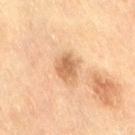Clinical impression: The lesion was photographed on a routine skin check and not biopsied; there is no pathology result. Acquisition and patient details: A region of skin cropped from a whole-body photographic capture, roughly 15 mm wide. An algorithmic analysis of the crop reported a mean CIELAB color near L≈57 a*≈19 b*≈34, about 11 CIELAB-L* units darker than the surrounding skin, and a lesion-to-skin contrast of about 7.5 (normalized; higher = more distinct). The analysis additionally found border irregularity of about 2.5 on a 0–10 scale and a peripheral color-asymmetry measure near 1. The analysis additionally found an automated nevus-likeness rating near 5 out of 100. A female patient aged around 55. From the right thigh. Captured under cross-polarized illumination. The lesion's longest dimension is about 3.5 mm.